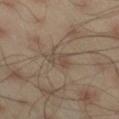Part of a total-body skin-imaging series; this lesion was reviewed on a skin check and was not flagged for biopsy.
Located on the right thigh.
Measured at roughly 3.5 mm in maximum diameter.
Cropped from a whole-body photographic skin survey; the tile spans about 15 mm.
The subject is a male in their mid- to late 40s.